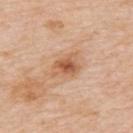Impression:
The lesion was photographed on a routine skin check and not biopsied; there is no pathology result.
Clinical summary:
The lesion is on the upper back. Longest diameter approximately 3.5 mm. This image is a 15 mm lesion crop taken from a total-body photograph. A male patient about 75 years old.